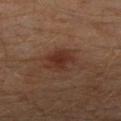The lesion was photographed on a routine skin check and not biopsied; there is no pathology result. Automated tile analysis of the lesion measured an area of roughly 8 mm², a shape eccentricity near 0.4, and a symmetry-axis asymmetry near 0.3. It also reported a normalized lesion–skin contrast near 7.5. And it measured a color-variation rating of about 2.5/10 and peripheral color asymmetry of about 1. The software also gave a nevus-likeness score of about 90/100 and a lesion-detection confidence of about 100/100. From the left leg. A male subject aged 28–32. Cropped from a total-body skin-imaging series; the visible field is about 15 mm. Imaged with cross-polarized lighting. About 3.5 mm across.